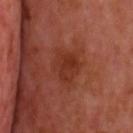Q: Was a biopsy performed?
A: catalogued during a skin exam; not biopsied
Q: How large is the lesion?
A: ≈4 mm
Q: What lighting was used for the tile?
A: cross-polarized
Q: What kind of image is this?
A: ~15 mm crop, total-body skin-cancer survey
Q: Automated lesion metrics?
A: a lesion color around L≈34 a*≈28 b*≈31 in CIELAB and a lesion-to-skin contrast of about 6.5 (normalized; higher = more distinct); a border-irregularity index near 3/10 and a within-lesion color-variation index near 3/10
Q: What are the patient's age and sex?
A: male, aged around 55
Q: What is the anatomic site?
A: the head or neck